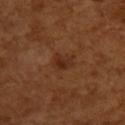This lesion was catalogued during total-body skin photography and was not selected for biopsy.
The recorded lesion diameter is about 2.5 mm.
Captured under cross-polarized illumination.
From the upper back.
A female subject, in their mid-50s.
A 15 mm crop from a total-body photograph taken for skin-cancer surveillance.